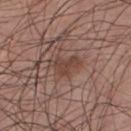Impression: Imaged during a routine full-body skin examination; the lesion was not biopsied and no histopathology is available. Acquisition and patient details: This image is a 15 mm lesion crop taken from a total-body photograph. The lesion is on the front of the torso. The recorded lesion diameter is about 4 mm. The tile uses white-light illumination. A male patient, approximately 30 years of age. The total-body-photography lesion software estimated border irregularity of about 5.5 on a 0–10 scale, internal color variation of about 2 on a 0–10 scale, and radial color variation of about 0.5. It also reported a nevus-likeness score of about 10/100.